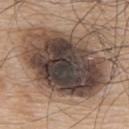Q: How large is the lesion?
A: ~10 mm (longest diameter)
Q: How was the tile lit?
A: white-light
Q: Lesion location?
A: the mid back
Q: Patient demographics?
A: male, aged 73–77
Q: What kind of image is this?
A: ~15 mm tile from a whole-body skin photo